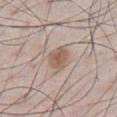Findings:
* follow-up — catalogued during a skin exam; not biopsied
* patient — male, aged around 70
* image — ~15 mm crop, total-body skin-cancer survey
* image-analysis metrics — a lesion area of about 6 mm², a shape eccentricity near 0.65, and a shape-asymmetry score of about 0.15 (0 = symmetric); roughly 10 lightness units darker than nearby skin and a lesion-to-skin contrast of about 7.5 (normalized; higher = more distinct); a border-irregularity rating of about 1.5/10 and a peripheral color-asymmetry measure near 1; a nevus-likeness score of about 90/100
* illumination — white-light
* size — ~3 mm (longest diameter)
* site — the chest